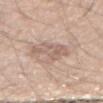Clinical impression:
The lesion was photographed on a routine skin check and not biopsied; there is no pathology result.
Acquisition and patient details:
Approximately 4 mm at its widest. The patient is a male in their mid-40s. The tile uses white-light illumination. A lesion tile, about 15 mm wide, cut from a 3D total-body photograph. Automated tile analysis of the lesion measured an average lesion color of about L≈61 a*≈16 b*≈25 (CIELAB), a lesion–skin lightness drop of about 8, and a normalized lesion–skin contrast near 5.5. And it measured a nevus-likeness score of about 0/100 and a lesion-detection confidence of about 85/100. On the right forearm.